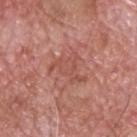Recorded during total-body skin imaging; not selected for excision or biopsy.
Captured under white-light illumination.
Located on the upper back.
About 4 mm across.
The subject is a male aged approximately 60.
Cropped from a whole-body photographic skin survey; the tile spans about 15 mm.